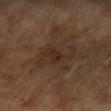Part of a total-body skin-imaging series; this lesion was reviewed on a skin check and was not flagged for biopsy.
A 15 mm close-up extracted from a 3D total-body photography capture.
This is a cross-polarized tile.
Located on the left forearm.
A female subject, in their 60s.
The total-body-photography lesion software estimated an eccentricity of roughly 0.65 and a shape-asymmetry score of about 0.45 (0 = symmetric). The analysis additionally found an average lesion color of about L≈24 a*≈14 b*≈21 (CIELAB) and roughly 5 lightness units darker than nearby skin. The software also gave border irregularity of about 7 on a 0–10 scale, a within-lesion color-variation index near 3/10, and radial color variation of about 1.